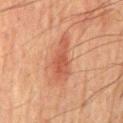Case summary:
* notes · no biopsy performed (imaged during a skin exam)
* lesion diameter · ≈6.5 mm
* site · the mid back
* subject · male, aged around 65
* tile lighting · cross-polarized
* acquisition · 15 mm crop, total-body photography